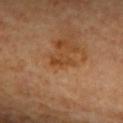Part of a total-body skin-imaging series; this lesion was reviewed on a skin check and was not flagged for biopsy.
Measured at roughly 2.5 mm in maximum diameter.
Imaged with cross-polarized lighting.
The patient is a male approximately 65 years of age.
The total-body-photography lesion software estimated a footprint of about 2.5 mm² and two-axis asymmetry of about 0.35. The software also gave about 8 CIELAB-L* units darker than the surrounding skin and a normalized border contrast of about 7. The analysis additionally found a border-irregularity index near 4.5/10, internal color variation of about 0 on a 0–10 scale, and radial color variation of about 0.
From the back.
Cropped from a whole-body photographic skin survey; the tile spans about 15 mm.